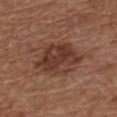{"biopsy_status": "not biopsied; imaged during a skin examination", "lighting": "white-light", "patient": {"sex": "male", "age_approx": 65}, "lesion_size": {"long_diameter_mm_approx": 6.5}, "image": {"source": "total-body photography crop", "field_of_view_mm": 15}, "site": "chest", "automated_metrics": {"area_mm2_approx": 20.0, "eccentricity": 0.7, "cielab_L": 37, "cielab_a": 21, "cielab_b": 26, "vs_skin_darker_L": 10.0, "vs_skin_contrast_norm": 8.5, "border_irregularity_0_10": 2.5, "color_variation_0_10": 5.0, "peripheral_color_asymmetry": 1.5, "nevus_likeness_0_100": 15, "lesion_detection_confidence_0_100": 100}}